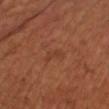Notes:
- notes — total-body-photography surveillance lesion; no biopsy
- lighting — cross-polarized
- subject — male, in their 60s
- image source — total-body-photography crop, ~15 mm field of view
- image-analysis metrics — an area of roughly 2.5 mm², a shape eccentricity near 0.9, and two-axis asymmetry of about 0.4; a border-irregularity index near 4.5/10, internal color variation of about 0 on a 0–10 scale, and a peripheral color-asymmetry measure near 0; a nevus-likeness score of about 0/100 and a lesion-detection confidence of about 100/100
- size — ~2.5 mm (longest diameter)
- anatomic site — the right upper arm